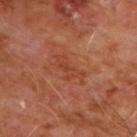Recorded during total-body skin imaging; not selected for excision or biopsy. A 15 mm close-up extracted from a 3D total-body photography capture. Located on the chest. A male subject aged around 60. An algorithmic analysis of the crop reported a mean CIELAB color near L≈41 a*≈27 b*≈33, a lesion–skin lightness drop of about 6, and a normalized border contrast of about 5. It also reported a border-irregularity rating of about 5.5/10, a within-lesion color-variation index near 1.5/10, and peripheral color asymmetry of about 0.5. It also reported an automated nevus-likeness rating near 0 out of 100 and a lesion-detection confidence of about 100/100. The tile uses cross-polarized illumination. The lesion's longest dimension is about 4 mm.